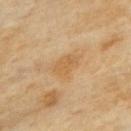This lesion was catalogued during total-body skin photography and was not selected for biopsy. A lesion tile, about 15 mm wide, cut from a 3D total-body photograph. About 4 mm across. The patient is a female roughly 60 years of age. Captured under cross-polarized illumination. On the upper back.